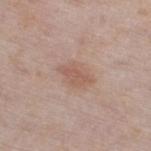Captured during whole-body skin photography for melanoma surveillance; the lesion was not biopsied.
The lesion's longest dimension is about 3.5 mm.
Imaged with white-light lighting.
Cropped from a total-body skin-imaging series; the visible field is about 15 mm.
The patient is a female aged approximately 60.
An algorithmic analysis of the crop reported a mean CIELAB color near L≈57 a*≈19 b*≈26, about 7 CIELAB-L* units darker than the surrounding skin, and a lesion-to-skin contrast of about 5.5 (normalized; higher = more distinct). And it measured an automated nevus-likeness rating near 50 out of 100 and a detector confidence of about 100 out of 100 that the crop contains a lesion.
The lesion is on the right thigh.